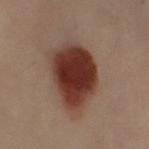Impression:
This lesion was catalogued during total-body skin photography and was not selected for biopsy.
Image and clinical context:
Approximately 6 mm at its widest. The lesion is on the right leg. A female patient aged 58 to 62. A 15 mm close-up tile from a total-body photography series done for melanoma screening. The lesion-visualizer software estimated a lesion area of about 23 mm², a shape eccentricity near 0.65, and a shape-asymmetry score of about 0.2 (0 = symmetric). The software also gave border irregularity of about 2 on a 0–10 scale, a color-variation rating of about 4/10, and radial color variation of about 1. And it measured a nevus-likeness score of about 100/100 and lesion-presence confidence of about 100/100.